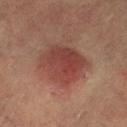biopsy_status: not biopsied; imaged during a skin examination
lesion_size:
  long_diameter_mm_approx: 5.0
lighting: cross-polarized
patient:
  sex: female
  age_approx: 60
site: leg
image:
  source: total-body photography crop
  field_of_view_mm: 15